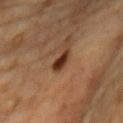notes: no biopsy performed (imaged during a skin exam) | patient: male, in their mid-80s or older | image source: ~15 mm crop, total-body skin-cancer survey | location: the chest | tile lighting: cross-polarized illumination | size: about 3 mm | automated metrics: a shape eccentricity near 0.85 and a shape-asymmetry score of about 0.3 (0 = symmetric); a border-irregularity rating of about 3/10, a within-lesion color-variation index near 1.5/10, and radial color variation of about 0.5; a classifier nevus-likeness of about 100/100 and a detector confidence of about 100 out of 100 that the crop contains a lesion.